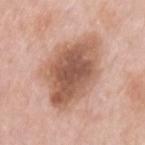notes: catalogued during a skin exam; not biopsied